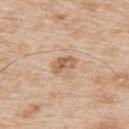Captured during whole-body skin photography for melanoma surveillance; the lesion was not biopsied.
The lesion-visualizer software estimated an area of roughly 4.5 mm² and a symmetry-axis asymmetry near 0.4. The analysis additionally found a lesion color around L≈60 a*≈19 b*≈32 in CIELAB, a lesion–skin lightness drop of about 11, and a normalized lesion–skin contrast near 7.
The lesion is located on the upper back.
A male subject, approximately 65 years of age.
This is a white-light tile.
Cropped from a total-body skin-imaging series; the visible field is about 15 mm.
The lesion's longest dimension is about 3 mm.